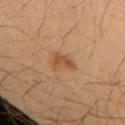{"image": {"source": "total-body photography crop", "field_of_view_mm": 15}, "automated_metrics": {"area_mm2_approx": 4.0, "eccentricity": 0.85, "shape_asymmetry": 0.45, "cielab_L": 40, "cielab_a": 17, "cielab_b": 30, "vs_skin_darker_L": 7.0, "vs_skin_contrast_norm": 6.5}, "lesion_size": {"long_diameter_mm_approx": 3.5}, "site": "arm", "lighting": "cross-polarized", "patient": {"sex": "female", "age_approx": 40}}